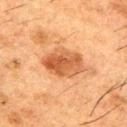Acquisition and patient details:
A roughly 15 mm field-of-view crop from a total-body skin photograph. Longest diameter approximately 5.5 mm. Automated tile analysis of the lesion measured an area of roughly 14 mm², a shape eccentricity near 0.8, and a shape-asymmetry score of about 0.15 (0 = symmetric). And it measured a mean CIELAB color near L≈46 a*≈23 b*≈34 and a lesion–skin lightness drop of about 11. It also reported border irregularity of about 2 on a 0–10 scale, a within-lesion color-variation index near 5.5/10, and radial color variation of about 2. Located on the right upper arm. A male patient, aged 48 to 52.